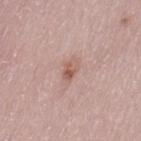follow-up: total-body-photography surveillance lesion; no biopsy
TBP lesion metrics: an area of roughly 3.5 mm², an eccentricity of roughly 0.8, and two-axis asymmetry of about 0.2; a border-irregularity index near 2/10 and radial color variation of about 2.5
anatomic site: the left thigh
subject: female, in their 30s
image source: total-body-photography crop, ~15 mm field of view
tile lighting: white-light